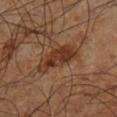| key | value |
|---|---|
| notes | imaged on a skin check; not biopsied |
| image-analysis metrics | an eccentricity of roughly 0.85 and a symmetry-axis asymmetry near 0.25; an average lesion color of about L≈34 a*≈20 b*≈28 (CIELAB) and a lesion–skin lightness drop of about 10; border irregularity of about 3 on a 0–10 scale, a within-lesion color-variation index near 3.5/10, and radial color variation of about 1 |
| subject | male, approximately 60 years of age |
| acquisition | ~15 mm tile from a whole-body skin photo |
| size | ≈5.5 mm |
| anatomic site | the left lower leg |
| illumination | cross-polarized illumination |